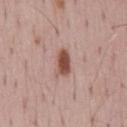* follow-up — catalogued during a skin exam; not biopsied
* image — 15 mm crop, total-body photography
* subject — male, aged around 70
* location — the mid back
* size — ~3.5 mm (longest diameter)
* automated metrics — a footprint of about 6 mm², an outline eccentricity of about 0.85 (0 = round, 1 = elongated), and a symmetry-axis asymmetry near 0.25
* lighting — white-light